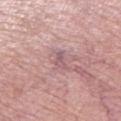Notes:
* biopsy status: imaged on a skin check; not biopsied
* diameter: ~2.5 mm (longest diameter)
* anatomic site: the left thigh
* imaging modality: total-body-photography crop, ~15 mm field of view
* patient: female, in their 70s